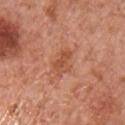biopsy status: catalogued during a skin exam; not biopsied
illumination: white-light illumination
anatomic site: the chest
lesion size: about 3.5 mm
imaging modality: ~15 mm crop, total-body skin-cancer survey
automated metrics: a footprint of about 5 mm², an outline eccentricity of about 0.8 (0 = round, 1 = elongated), and two-axis asymmetry of about 0.35; a within-lesion color-variation index near 3/10 and radial color variation of about 1; an automated nevus-likeness rating near 5 out of 100 and lesion-presence confidence of about 100/100
subject: male, approximately 65 years of age